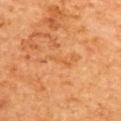No biopsy was performed on this lesion — it was imaged during a full skin examination and was not determined to be concerning. Located on the upper back. The patient is female. A 15 mm close-up tile from a total-body photography series done for melanoma screening. Measured at roughly 3 mm in maximum diameter.